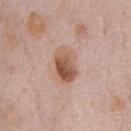| field | value |
|---|---|
| follow-up | catalogued during a skin exam; not biopsied |
| subject | male, aged 58 to 62 |
| automated lesion analysis | a lesion color around L≈56 a*≈20 b*≈30 in CIELAB, about 12 CIELAB-L* units darker than the surrounding skin, and a normalized lesion–skin contrast near 9 |
| location | the chest |
| image source | total-body-photography crop, ~15 mm field of view |
| lesion size | ~4.5 mm (longest diameter) |
| illumination | white-light illumination |